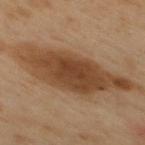Recorded during total-body skin imaging; not selected for excision or biopsy.
About 12 mm across.
This image is a 15 mm lesion crop taken from a total-body photograph.
An algorithmic analysis of the crop reported a symmetry-axis asymmetry near 0.25. And it measured border irregularity of about 4 on a 0–10 scale, internal color variation of about 5 on a 0–10 scale, and a peripheral color-asymmetry measure near 1.5. The analysis additionally found an automated nevus-likeness rating near 85 out of 100.
On the mid back.
A male patient, in their 50s.